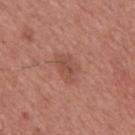Part of a total-body skin-imaging series; this lesion was reviewed on a skin check and was not flagged for biopsy. A male patient approximately 45 years of age. Approximately 3.5 mm at its widest. Automated tile analysis of the lesion measured a footprint of about 6 mm², an eccentricity of roughly 0.8, and a shape-asymmetry score of about 0.35 (0 = symmetric). The analysis additionally found an average lesion color of about L≈50 a*≈24 b*≈28 (CIELAB), a lesion–skin lightness drop of about 7, and a normalized lesion–skin contrast near 5.5. The analysis additionally found border irregularity of about 4 on a 0–10 scale and a peripheral color-asymmetry measure near 1. The lesion is on the chest. A close-up tile cropped from a whole-body skin photograph, about 15 mm across. The tile uses white-light illumination.